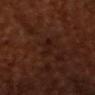biopsy status = no biopsy performed (imaged during a skin exam)
acquisition = ~15 mm tile from a whole-body skin photo
tile lighting = cross-polarized
lesion size = ≈3.5 mm
site = the chest
subject = male, roughly 65 years of age